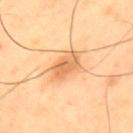| feature | finding |
|---|---|
| biopsy status | no biopsy performed (imaged during a skin exam) |
| body site | the upper back |
| subject | male, roughly 65 years of age |
| acquisition | ~15 mm crop, total-body skin-cancer survey |